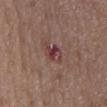Acquisition and patient details: A 15 mm crop from a total-body photograph taken for skin-cancer surveillance. From the mid back. Automated tile analysis of the lesion measured an automated nevus-likeness rating near 0 out of 100 and lesion-presence confidence of about 100/100. A male patient in their 50s.